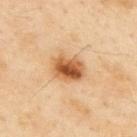Impression: Part of a total-body skin-imaging series; this lesion was reviewed on a skin check and was not flagged for biopsy. Acquisition and patient details: Approximately 4.5 mm at its widest. A male subject, in their mid- to late 50s. The lesion is located on the upper back. A 15 mm close-up extracted from a 3D total-body photography capture. Imaged with cross-polarized lighting.